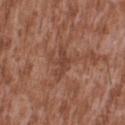Q: Was a biopsy performed?
A: imaged on a skin check; not biopsied
Q: What are the patient's age and sex?
A: male, aged approximately 45
Q: Where on the body is the lesion?
A: the back
Q: How was the tile lit?
A: white-light illumination
Q: How large is the lesion?
A: ≈3.5 mm
Q: What is the imaging modality?
A: 15 mm crop, total-body photography